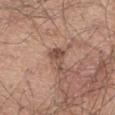Assessment:
Imaged during a routine full-body skin examination; the lesion was not biopsied and no histopathology is available.
Acquisition and patient details:
A male patient aged around 40. A close-up tile cropped from a whole-body skin photograph, about 15 mm across. Approximately 3.5 mm at its widest. From the left thigh. The tile uses white-light illumination. Automated tile analysis of the lesion measured a within-lesion color-variation index near 2/10 and a peripheral color-asymmetry measure near 0.5.